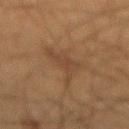Q: Is there a histopathology result?
A: imaged on a skin check; not biopsied
Q: Automated lesion metrics?
A: an automated nevus-likeness rating near 0 out of 100
Q: Where on the body is the lesion?
A: the back
Q: What lighting was used for the tile?
A: cross-polarized
Q: How was this image acquired?
A: 15 mm crop, total-body photography
Q: Patient demographics?
A: male, aged 63 to 67